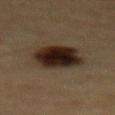Findings:
– acquisition · 15 mm crop, total-body photography
– site · the abdomen
– size · about 5 mm
– patient · male, approximately 85 years of age or older